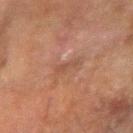Findings:
• notes · catalogued during a skin exam; not biopsied
• image source · 15 mm crop, total-body photography
• patient · male, about 65 years old
• location · the left forearm
• image-analysis metrics · a lesion area of about 3 mm², an eccentricity of roughly 0.9, and two-axis asymmetry of about 0.35; an automated nevus-likeness rating near 0 out of 100
• size · about 3 mm